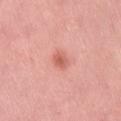Notes:
– notes — total-body-photography surveillance lesion; no biopsy
– TBP lesion metrics — a border-irregularity index near 2/10, a color-variation rating of about 2.5/10, and radial color variation of about 1; a nevus-likeness score of about 95/100 and a detector confidence of about 100 out of 100 that the crop contains a lesion
– lighting — white-light
– anatomic site — the right thigh
– patient — female, approximately 50 years of age
– image source — ~15 mm tile from a whole-body skin photo
– lesion size — about 2.5 mm